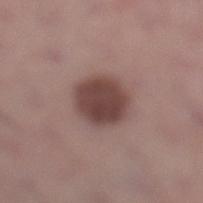TBP lesion metrics: a footprint of about 13 mm², an outline eccentricity of about 0.55 (0 = round, 1 = elongated), and two-axis asymmetry of about 0.1; border irregularity of about 1 on a 0–10 scale and radial color variation of about 1 | anatomic site: the left lower leg | diameter: ~4.5 mm (longest diameter) | subject: male, aged 33–37 | illumination: white-light illumination | imaging modality: ~15 mm crop, total-body skin-cancer survey.